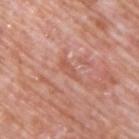The lesion was tiled from a total-body skin photograph and was not biopsied.
From the upper back.
This is a white-light tile.
A 15 mm crop from a total-body photograph taken for skin-cancer surveillance.
The patient is a male aged 68–72.
The recorded lesion diameter is about 3.5 mm.